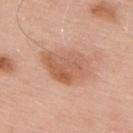Q: Is there a histopathology result?
A: total-body-photography surveillance lesion; no biopsy
Q: Lesion size?
A: ~6 mm (longest diameter)
Q: Patient demographics?
A: male, aged around 55
Q: Lesion location?
A: the upper back
Q: What did automated image analysis measure?
A: an area of roughly 16 mm², an outline eccentricity of about 0.8 (0 = round, 1 = elongated), and two-axis asymmetry of about 0.25; a lesion–skin lightness drop of about 10 and a lesion-to-skin contrast of about 7 (normalized; higher = more distinct); a border-irregularity index near 2.5/10 and a within-lesion color-variation index near 4.5/10
Q: What is the imaging modality?
A: ~15 mm tile from a whole-body skin photo